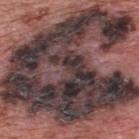Longest diameter approximately 16.5 mm. This is a white-light tile. The lesion is located on the upper back. This image is a 15 mm lesion crop taken from a total-body photograph. A male subject, about 70 years old. On excision, pathology confirmed a melanoma in situ.